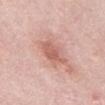No biopsy was performed on this lesion — it was imaged during a full skin examination and was not determined to be concerning.